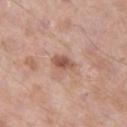<case>
  <biopsy_status>not biopsied; imaged during a skin examination</biopsy_status>
  <patient>
    <sex>male</sex>
    <age_approx>55</age_approx>
  </patient>
  <lesion_size>
    <long_diameter_mm_approx>3.0</long_diameter_mm_approx>
  </lesion_size>
  <site>left thigh</site>
  <lighting>white-light</lighting>
  <image>
    <source>total-body photography crop</source>
    <field_of_view_mm>15</field_of_view_mm>
  </image>
</case>